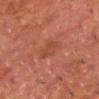Clinical impression:
Recorded during total-body skin imaging; not selected for excision or biopsy.
Acquisition and patient details:
A male patient in their 80s. A lesion tile, about 15 mm wide, cut from a 3D total-body photograph. On the head or neck.